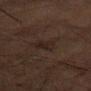Imaged during a routine full-body skin examination; the lesion was not biopsied and no histopathology is available. A 15 mm close-up extracted from a 3D total-body photography capture. The lesion is on the right thigh. A male patient, in their mid- to late 80s or older. Longest diameter approximately 3.5 mm. Captured under cross-polarized illumination.